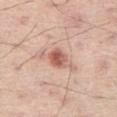Clinical impression: The lesion was tiled from a total-body skin photograph and was not biopsied. Context: Captured under white-light illumination. From the right thigh. Longest diameter approximately 2.5 mm. A male patient aged around 55. Automated tile analysis of the lesion measured a lesion area of about 5 mm². It also reported a border-irregularity index near 1.5/10 and a peripheral color-asymmetry measure near 1.5. The software also gave a nevus-likeness score of about 90/100. A lesion tile, about 15 mm wide, cut from a 3D total-body photograph.